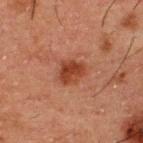Assessment: The lesion was photographed on a routine skin check and not biopsied; there is no pathology result. Image and clinical context: A male patient, aged 48–52. This is a cross-polarized tile. The lesion is on the upper back. The lesion's longest dimension is about 3 mm. Cropped from a total-body skin-imaging series; the visible field is about 15 mm.